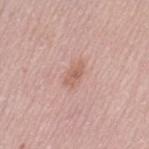This lesion was catalogued during total-body skin photography and was not selected for biopsy.
The lesion is located on the left thigh.
A female patient in their mid-60s.
Imaged with white-light lighting.
A roughly 15 mm field-of-view crop from a total-body skin photograph.